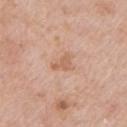Findings:
– biopsy status · no biopsy performed (imaged during a skin exam)
– image-analysis metrics · a mean CIELAB color near L≈61 a*≈21 b*≈33, roughly 8 lightness units darker than nearby skin, and a lesion-to-skin contrast of about 6 (normalized; higher = more distinct); border irregularity of about 6 on a 0–10 scale, a color-variation rating of about 0.5/10, and a peripheral color-asymmetry measure near 0; a classifier nevus-likeness of about 0/100 and a detector confidence of about 100 out of 100 that the crop contains a lesion
– patient · male, aged around 65
– site · the left upper arm
– imaging modality · ~15 mm crop, total-body skin-cancer survey
– diameter · ~3 mm (longest diameter)
– lighting · white-light